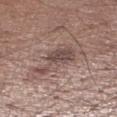The lesion was photographed on a routine skin check and not biopsied; there is no pathology result. A male subject roughly 55 years of age. The lesion-visualizer software estimated a lesion area of about 15 mm² and a shape eccentricity near 0.9. The analysis additionally found a lesion color around L≈49 a*≈16 b*≈21 in CIELAB and a normalized lesion–skin contrast near 6.5. It also reported border irregularity of about 6.5 on a 0–10 scale, a color-variation rating of about 5.5/10, and radial color variation of about 1.5. The software also gave a nevus-likeness score of about 0/100 and a detector confidence of about 100 out of 100 that the crop contains a lesion. A lesion tile, about 15 mm wide, cut from a 3D total-body photograph. Measured at roughly 6.5 mm in maximum diameter. From the right lower leg.